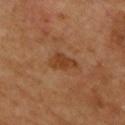Q: Is there a histopathology result?
A: no biopsy performed (imaged during a skin exam)
Q: Illumination type?
A: cross-polarized
Q: What did automated image analysis measure?
A: an area of roughly 5 mm², an outline eccentricity of about 0.85 (0 = round, 1 = elongated), and two-axis asymmetry of about 0.35; an average lesion color of about L≈39 a*≈23 b*≈34 (CIELAB) and roughly 8 lightness units darker than nearby skin; a lesion-detection confidence of about 100/100
Q: What is the anatomic site?
A: the chest
Q: How was this image acquired?
A: 15 mm crop, total-body photography
Q: What are the patient's age and sex?
A: male, approximately 65 years of age